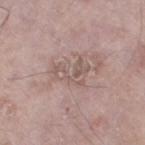workup — total-body-photography surveillance lesion; no biopsy
patient — male, approximately 65 years of age
diameter — about 4 mm
site — the left lower leg
automated lesion analysis — a shape eccentricity near 0.8; a mean CIELAB color near L≈55 a*≈16 b*≈22 and about 7 CIELAB-L* units darker than the surrounding skin; radial color variation of about 1.5; a nevus-likeness score of about 0/100 and a detector confidence of about 60 out of 100 that the crop contains a lesion
image source — 15 mm crop, total-body photography